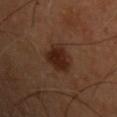<tbp_lesion>
<lesion_size>
  <long_diameter_mm_approx>3.5</long_diameter_mm_approx>
</lesion_size>
<automated_metrics>
  <area_mm2_approx>9.5</area_mm2_approx>
  <eccentricity>0.45</eccentricity>
  <shape_asymmetry>0.25</shape_asymmetry>
  <cielab_L>23</cielab_L>
  <cielab_a>19</cielab_a>
  <cielab_b>24</cielab_b>
  <vs_skin_darker_L>9.0</vs_skin_darker_L>
  <border_irregularity_0_10>2.5</border_irregularity_0_10>
  <peripheral_color_asymmetry>1.0</peripheral_color_asymmetry>
</automated_metrics>
<site>chest</site>
<patient>
  <sex>male</sex>
  <age_approx>55</age_approx>
</patient>
<image>
  <source>total-body photography crop</source>
  <field_of_view_mm>15</field_of_view_mm>
</image>
<lighting>cross-polarized</lighting>
</tbp_lesion>